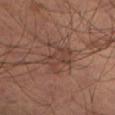Impression: This lesion was catalogued during total-body skin photography and was not selected for biopsy. Context: The lesion is located on the left thigh. A male patient aged approximately 65. Captured under cross-polarized illumination. The recorded lesion diameter is about 4.5 mm. A 15 mm crop from a total-body photograph taken for skin-cancer surveillance.